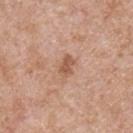| feature | finding |
|---|---|
| biopsy status | total-body-photography surveillance lesion; no biopsy |
| image-analysis metrics | a footprint of about 4 mm², an eccentricity of roughly 0.8, and a shape-asymmetry score of about 0.25 (0 = symmetric); a mean CIELAB color near L≈56 a*≈22 b*≈31 and about 10 CIELAB-L* units darker than the surrounding skin |
| image | 15 mm crop, total-body photography |
| subject | male, in their mid- to late 60s |
| location | the chest |